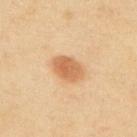Part of a total-body skin-imaging series; this lesion was reviewed on a skin check and was not flagged for biopsy. Imaged with cross-polarized lighting. Located on the back. A female patient aged 58–62. Automated tile analysis of the lesion measured an area of roughly 7.5 mm², an outline eccentricity of about 0.8 (0 = round, 1 = elongated), and two-axis asymmetry of about 0.15. The analysis additionally found a mean CIELAB color near L≈57 a*≈21 b*≈36, a lesion–skin lightness drop of about 11, and a lesion-to-skin contrast of about 8 (normalized; higher = more distinct). The software also gave lesion-presence confidence of about 100/100. About 4 mm across. A 15 mm close-up extracted from a 3D total-body photography capture.